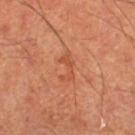{
  "biopsy_status": "not biopsied; imaged during a skin examination",
  "site": "right lower leg",
  "image": {
    "source": "total-body photography crop",
    "field_of_view_mm": 15
  },
  "patient": {
    "sex": "male",
    "age_approx": 65
  },
  "lighting": "cross-polarized",
  "lesion_size": {
    "long_diameter_mm_approx": 3.5
  }
}